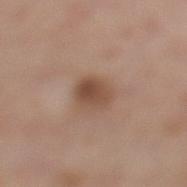Q: Was this lesion biopsied?
A: total-body-photography surveillance lesion; no biopsy
Q: What is the lesion's diameter?
A: about 3.5 mm
Q: How was the tile lit?
A: white-light illumination
Q: What is the anatomic site?
A: the left lower leg
Q: What are the patient's age and sex?
A: female, aged approximately 55
Q: What kind of image is this?
A: ~15 mm tile from a whole-body skin photo
Q: Automated lesion metrics?
A: an outline eccentricity of about 0.65 (0 = round, 1 = elongated) and a shape-asymmetry score of about 0.15 (0 = symmetric); an automated nevus-likeness rating near 70 out of 100 and lesion-presence confidence of about 100/100